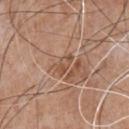Assessment: The lesion was photographed on a routine skin check and not biopsied; there is no pathology result. Clinical summary: Measured at roughly 3 mm in maximum diameter. From the chest. Cropped from a whole-body photographic skin survey; the tile spans about 15 mm. The tile uses white-light illumination. A male patient, roughly 65 years of age.